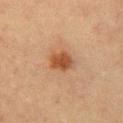<case>
<biopsy_status>not biopsied; imaged during a skin examination</biopsy_status>
<patient>
  <sex>female</sex>
  <age_approx>55</age_approx>
</patient>
<lesion_size>
  <long_diameter_mm_approx>3.0</long_diameter_mm_approx>
</lesion_size>
<automated_metrics>
  <vs_skin_darker_L>10.0</vs_skin_darker_L>
  <border_irregularity_0_10>1.5</border_irregularity_0_10>
  <color_variation_0_10>2.5</color_variation_0_10>
  <peripheral_color_asymmetry>1.0</peripheral_color_asymmetry>
</automated_metrics>
<image>
  <source>total-body photography crop</source>
  <field_of_view_mm>15</field_of_view_mm>
</image>
<lighting>cross-polarized</lighting>
<site>chest</site>
</case>